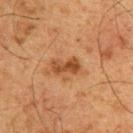Assessment:
This lesion was catalogued during total-body skin photography and was not selected for biopsy.
Clinical summary:
A male patient, aged 58 to 62. On the upper back. Cropped from a total-body skin-imaging series; the visible field is about 15 mm. Captured under cross-polarized illumination.